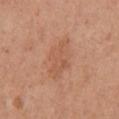workup: no biopsy performed (imaged during a skin exam)
illumination: white-light illumination
patient: female, aged approximately 65
lesion diameter: ~5 mm (longest diameter)
imaging modality: 15 mm crop, total-body photography
location: the chest